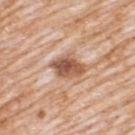Q: Is there a histopathology result?
A: no biopsy performed (imaged during a skin exam)
Q: How large is the lesion?
A: ~4 mm (longest diameter)
Q: How was the tile lit?
A: white-light
Q: Patient demographics?
A: male, roughly 80 years of age
Q: Lesion location?
A: the upper back
Q: What is the imaging modality?
A: 15 mm crop, total-body photography